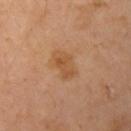• follow-up — imaged on a skin check; not biopsied
• automated lesion analysis — an outline eccentricity of about 0.75 (0 = round, 1 = elongated) and two-axis asymmetry of about 0.2; a lesion color around L≈53 a*≈21 b*≈37 in CIELAB, a lesion–skin lightness drop of about 7, and a normalized lesion–skin contrast near 6; a border-irregularity index near 2.5/10, a within-lesion color-variation index near 3/10, and a peripheral color-asymmetry measure near 1
• anatomic site — the arm
• diameter — about 3.5 mm
• image — ~15 mm tile from a whole-body skin photo
• patient — female, aged around 45